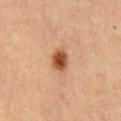The subject is a female aged approximately 45. The lesion's longest dimension is about 3 mm. Located on the lower back. The lesion-visualizer software estimated a lesion color around L≈51 a*≈25 b*≈35 in CIELAB, about 15 CIELAB-L* units darker than the surrounding skin, and a normalized lesion–skin contrast near 10.5. The tile uses cross-polarized illumination. A 15 mm close-up tile from a total-body photography series done for melanoma screening.